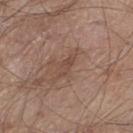patient: male, in their 80s
image source: total-body-photography crop, ~15 mm field of view
automated lesion analysis: a footprint of about 2.5 mm² and a shape eccentricity near 0.9; a color-variation rating of about 0/10 and peripheral color asymmetry of about 0
anatomic site: the left thigh